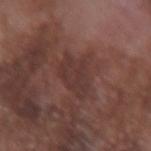Captured during whole-body skin photography for melanoma surveillance; the lesion was not biopsied.
The lesion is located on the right forearm.
A close-up tile cropped from a whole-body skin photograph, about 15 mm across.
An algorithmic analysis of the crop reported a border-irregularity rating of about 4/10, internal color variation of about 2.5 on a 0–10 scale, and radial color variation of about 1. It also reported a classifier nevus-likeness of about 0/100 and a detector confidence of about 100 out of 100 that the crop contains a lesion.
Approximately 4.5 mm at its widest.
The subject is a male aged around 75.
The tile uses white-light illumination.